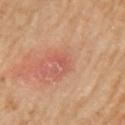Image and clinical context:
About 1 mm across. The lesion is on the left upper arm. The tile uses cross-polarized illumination. A region of skin cropped from a whole-body photographic capture, roughly 15 mm wide. A female patient, aged 68–72.